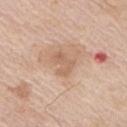image-analysis metrics — a footprint of about 5.5 mm², an eccentricity of roughly 0.75, and two-axis asymmetry of about 0.3; a lesion–skin lightness drop of about 8 and a normalized border contrast of about 5.5; a border-irregularity rating of about 4/10, a color-variation rating of about 2.5/10, and peripheral color asymmetry of about 1; a classifier nevus-likeness of about 0/100 and lesion-presence confidence of about 100/100 | tile lighting — white-light illumination | diameter — about 3.5 mm | patient — male, aged around 75 | acquisition — 15 mm crop, total-body photography | body site — the right upper arm.